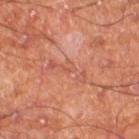Impression: No biopsy was performed on this lesion — it was imaged during a full skin examination and was not determined to be concerning. Acquisition and patient details: Captured under cross-polarized illumination. A male subject, about 60 years old. Cropped from a whole-body photographic skin survey; the tile spans about 15 mm. On the right thigh. Approximately 1.5 mm at its widest.